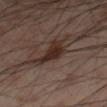Q: Was this lesion biopsied?
A: imaged on a skin check; not biopsied
Q: Lesion location?
A: the right thigh
Q: What lighting was used for the tile?
A: cross-polarized illumination
Q: What did automated image analysis measure?
A: an area of roughly 3.5 mm², an eccentricity of roughly 0.85, and a shape-asymmetry score of about 0.3 (0 = symmetric); a within-lesion color-variation index near 1.5/10; an automated nevus-likeness rating near 90 out of 100
Q: How was this image acquired?
A: ~15 mm crop, total-body skin-cancer survey
Q: What are the patient's age and sex?
A: male, about 55 years old